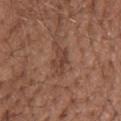Q: Lesion location?
A: the head or neck
Q: Automated lesion metrics?
A: a border-irregularity rating of about 4/10, internal color variation of about 2.5 on a 0–10 scale, and peripheral color asymmetry of about 0.5
Q: Patient demographics?
A: male, aged 73 to 77
Q: What lighting was used for the tile?
A: white-light
Q: What is the lesion's diameter?
A: ~3.5 mm (longest diameter)
Q: What kind of image is this?
A: ~15 mm tile from a whole-body skin photo